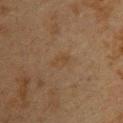biopsy status: total-body-photography surveillance lesion; no biopsy
illumination: cross-polarized
image-analysis metrics: roughly 3 lightness units darker than nearby skin and a lesion-to-skin contrast of about 4.5 (normalized; higher = more distinct)
location: the upper back
image source: 15 mm crop, total-body photography
patient: female, aged approximately 40